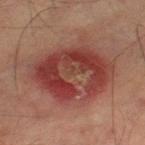Notes:
– lesion diameter · ≈8.5 mm
– location · the leg
– imaging modality · 15 mm crop, total-body photography
– patient · male, in their mid-70s